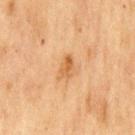Assessment: Recorded during total-body skin imaging; not selected for excision or biopsy. Background: From the chest. The lesion-visualizer software estimated an average lesion color of about L≈49 a*≈19 b*≈35 (CIELAB) and a normalized lesion–skin contrast near 6.5. The analysis additionally found a border-irregularity index near 3/10, a within-lesion color-variation index near 3/10, and peripheral color asymmetry of about 1. A lesion tile, about 15 mm wide, cut from a 3D total-body photograph. A male subject, in their mid-70s.